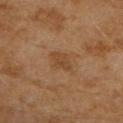The total-body-photography lesion software estimated an area of roughly 4 mm² and a shape eccentricity near 0.8. The software also gave a color-variation rating of about 1.5/10 and a peripheral color-asymmetry measure near 0.5. It also reported a nevus-likeness score of about 10/100 and a detector confidence of about 100 out of 100 that the crop contains a lesion. Located on the arm. A roughly 15 mm field-of-view crop from a total-body skin photograph. Approximately 3 mm at its widest. A female subject, aged 58 to 62.